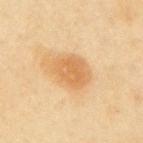follow-up: catalogued during a skin exam; not biopsied
subject: female, approximately 60 years of age
tile lighting: cross-polarized
size: ≈4.5 mm
acquisition: ~15 mm crop, total-body skin-cancer survey
body site: the mid back
automated metrics: a lesion color around L≈61 a*≈19 b*≈40 in CIELAB and a normalized border contrast of about 6.5; a detector confidence of about 100 out of 100 that the crop contains a lesion